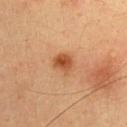Context: A female patient aged approximately 30. The recorded lesion diameter is about 2.5 mm. The lesion is on the back. Imaged with cross-polarized lighting. A close-up tile cropped from a whole-body skin photograph, about 15 mm across.